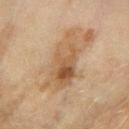Notes:
* size · about 6.5 mm
* acquisition · 15 mm crop, total-body photography
* body site · the right forearm
* lighting · cross-polarized illumination
* subject · female, aged 58–62
* TBP lesion metrics · an area of roughly 17 mm², a shape eccentricity near 0.85, and a symmetry-axis asymmetry near 0.25; a border-irregularity index near 4.5/10, internal color variation of about 8.5 on a 0–10 scale, and radial color variation of about 3; a classifier nevus-likeness of about 0/100 and lesion-presence confidence of about 100/100